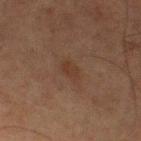Part of a total-body skin-imaging series; this lesion was reviewed on a skin check and was not flagged for biopsy.
The subject is a male roughly 75 years of age.
From the left thigh.
About 2.5 mm across.
Cropped from a total-body skin-imaging series; the visible field is about 15 mm.